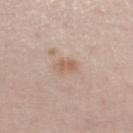Notes:
* notes · imaged on a skin check; not biopsied
* lesion diameter · ~2.5 mm (longest diameter)
* automated lesion analysis · an average lesion color of about L≈61 a*≈18 b*≈29 (CIELAB)
* patient · male, aged 58 to 62
* illumination · white-light illumination
* location · the right thigh
* acquisition · total-body-photography crop, ~15 mm field of view